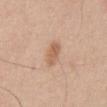Clinical impression: The lesion was tiled from a total-body skin photograph and was not biopsied. Acquisition and patient details: This is a white-light tile. A roughly 15 mm field-of-view crop from a total-body skin photograph. Automated image analysis of the tile measured an area of roughly 5.5 mm², an eccentricity of roughly 0.8, and a shape-asymmetry score of about 0.2 (0 = symmetric). The software also gave an average lesion color of about L≈61 a*≈20 b*≈33 (CIELAB), a lesion–skin lightness drop of about 9, and a normalized lesion–skin contrast near 6.5. The software also gave peripheral color asymmetry of about 1. And it measured a detector confidence of about 100 out of 100 that the crop contains a lesion. Located on the abdomen. A male subject in their mid- to late 60s.